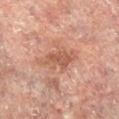Imaged during a routine full-body skin examination; the lesion was not biopsied and no histopathology is available. Automated tile analysis of the lesion measured border irregularity of about 4.5 on a 0–10 scale, internal color variation of about 2.5 on a 0–10 scale, and peripheral color asymmetry of about 1. From the left leg. Longest diameter approximately 4.5 mm. A female subject aged 78 to 82. A roughly 15 mm field-of-view crop from a total-body skin photograph.